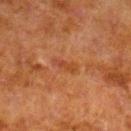Q: Is there a histopathology result?
A: imaged on a skin check; not biopsied
Q: Who is the patient?
A: male, aged 78 to 82
Q: What is the imaging modality?
A: 15 mm crop, total-body photography
Q: Automated lesion metrics?
A: a mean CIELAB color near L≈36 a*≈23 b*≈32, roughly 6 lightness units darker than nearby skin, and a normalized border contrast of about 5.5
Q: How large is the lesion?
A: about 3 mm
Q: Lesion location?
A: the leg